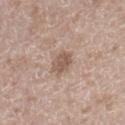<tbp_lesion>
<site>right lower leg</site>
<automated_metrics>
  <cielab_L>55</cielab_L>
  <cielab_a>17</cielab_a>
  <cielab_b>25</cielab_b>
  <vs_skin_darker_L>10.0</vs_skin_darker_L>
  <vs_skin_contrast_norm>7.0</vs_skin_contrast_norm>
  <border_irregularity_0_10>1.5</border_irregularity_0_10>
  <color_variation_0_10>2.5</color_variation_0_10>
  <peripheral_color_asymmetry>1.0</peripheral_color_asymmetry>
  <lesion_detection_confidence_0_100>100</lesion_detection_confidence_0_100>
</automated_metrics>
<lesion_size>
  <long_diameter_mm_approx>3.0</long_diameter_mm_approx>
</lesion_size>
<patient>
  <sex>male</sex>
  <age_approx>50</age_approx>
</patient>
<image>
  <source>total-body photography crop</source>
  <field_of_view_mm>15</field_of_view_mm>
</image>
<lighting>white-light</lighting>
</tbp_lesion>